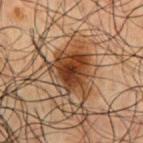The lesion was tiled from a total-body skin photograph and was not biopsied.
A 15 mm close-up tile from a total-body photography series done for melanoma screening.
A male patient about 50 years old.
The lesion is on the abdomen.